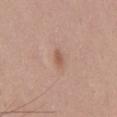Case summary:
* biopsy status · no biopsy performed (imaged during a skin exam)
* site · the chest
* illumination · white-light illumination
* automated lesion analysis · an area of roughly 2.5 mm² and a shape-asymmetry score of about 0.3 (0 = symmetric); a lesion–skin lightness drop of about 9; a classifier nevus-likeness of about 10/100 and a lesion-detection confidence of about 100/100
* size · ≈2.5 mm
* subject · male, approximately 50 years of age
* image · 15 mm crop, total-body photography